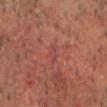This image is a 15 mm lesion crop taken from a total-body photograph.
The lesion is on the head or neck.
The patient is a male aged around 65.
Captured under cross-polarized illumination.